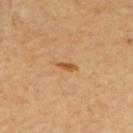<lesion>
<biopsy_status>not biopsied; imaged during a skin examination</biopsy_status>
<automated_metrics>
  <area_mm2_approx>2.5</area_mm2_approx>
  <eccentricity>0.9</eccentricity>
  <shape_asymmetry>0.25</shape_asymmetry>
  <cielab_L>55</cielab_L>
  <cielab_a>22</cielab_a>
  <cielab_b>42</cielab_b>
  <vs_skin_darker_L>10.0</vs_skin_darker_L>
  <vs_skin_contrast_norm>7.5</vs_skin_contrast_norm>
  <border_irregularity_0_10>2.5</border_irregularity_0_10>
  <color_variation_0_10>0.0</color_variation_0_10>
  <nevus_likeness_0_100>70</nevus_likeness_0_100>
  <lesion_detection_confidence_0_100>100</lesion_detection_confidence_0_100>
</automated_metrics>
<lesion_size>
  <long_diameter_mm_approx>2.5</long_diameter_mm_approx>
</lesion_size>
<site>upper back</site>
<lighting>cross-polarized</lighting>
<image>
  <source>total-body photography crop</source>
  <field_of_view_mm>15</field_of_view_mm>
</image>
<patient>
  <age_approx>60</age_approx>
</patient>
</lesion>